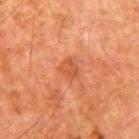Clinical impression: Captured during whole-body skin photography for melanoma surveillance; the lesion was not biopsied. Acquisition and patient details: A male subject aged around 80. A lesion tile, about 15 mm wide, cut from a 3D total-body photograph. Approximately 2.5 mm at its widest. On the right upper arm.